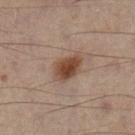<tbp_lesion>
<biopsy_status>not biopsied; imaged during a skin examination</biopsy_status>
<patient>
  <sex>male</sex>
  <age_approx>50</age_approx>
</patient>
<lighting>cross-polarized</lighting>
<lesion_size>
  <long_diameter_mm_approx>4.0</long_diameter_mm_approx>
</lesion_size>
<site>left leg</site>
<image>
  <source>total-body photography crop</source>
  <field_of_view_mm>15</field_of_view_mm>
</image>
<automated_metrics>
  <area_mm2_approx>8.5</area_mm2_approx>
  <nevus_likeness_0_100>100</nevus_likeness_0_100>
  <lesion_detection_confidence_0_100>100</lesion_detection_confidence_0_100>
</automated_metrics>
</tbp_lesion>